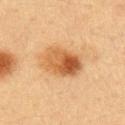Captured during whole-body skin photography for melanoma surveillance; the lesion was not biopsied. A lesion tile, about 15 mm wide, cut from a 3D total-body photograph. Captured under cross-polarized illumination. Measured at roughly 5 mm in maximum diameter. An algorithmic analysis of the crop reported border irregularity of about 1.5 on a 0–10 scale and peripheral color asymmetry of about 4. The lesion is located on the left upper arm. A male patient in their mid-50s.